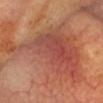biopsy status = total-body-photography surveillance lesion; no biopsy
lesion diameter = ≈11.5 mm
site = the head or neck
subject = male, roughly 60 years of age
image source = ~15 mm tile from a whole-body skin photo
image-analysis metrics = border irregularity of about 10 on a 0–10 scale and radial color variation of about 2
tile lighting = cross-polarized